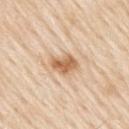Impression: The lesion was photographed on a routine skin check and not biopsied; there is no pathology result.